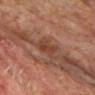| field | value |
|---|---|
| workup | catalogued during a skin exam; not biopsied |
| subject | male, roughly 65 years of age |
| imaging modality | ~15 mm tile from a whole-body skin photo |
| lesion diameter | ~3 mm (longest diameter) |
| site | the chest |
| tile lighting | cross-polarized illumination |